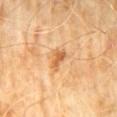biopsy status = total-body-photography surveillance lesion; no biopsy | image = ~15 mm crop, total-body skin-cancer survey | anatomic site = the abdomen | patient = male, aged 58–62 | size = about 3 mm.